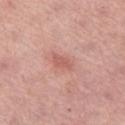{"biopsy_status": "not biopsied; imaged during a skin examination", "patient": {"sex": "female", "age_approx": 45}, "lesion_size": {"long_diameter_mm_approx": 2.5}, "image": {"source": "total-body photography crop", "field_of_view_mm": 15}, "site": "leg"}